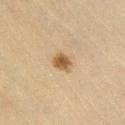Part of a total-body skin-imaging series; this lesion was reviewed on a skin check and was not flagged for biopsy. This is a cross-polarized tile. A female subject, aged 38–42. Cropped from a total-body skin-imaging series; the visible field is about 15 mm. An algorithmic analysis of the crop reported an outline eccentricity of about 0.6 (0 = round, 1 = elongated) and a shape-asymmetry score of about 0.2 (0 = symmetric). The software also gave about 11 CIELAB-L* units darker than the surrounding skin and a lesion-to-skin contrast of about 9 (normalized; higher = more distinct). The analysis additionally found peripheral color asymmetry of about 1. The analysis additionally found an automated nevus-likeness rating near 95 out of 100 and lesion-presence confidence of about 100/100. Located on the right lower leg.